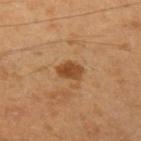Imaged during a routine full-body skin examination; the lesion was not biopsied and no histopathology is available.